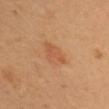Imaged during a routine full-body skin examination; the lesion was not biopsied and no histopathology is available. A female patient, aged 38–42. Captured under cross-polarized illumination. Cropped from a whole-body photographic skin survey; the tile spans about 15 mm. Longest diameter approximately 4 mm. Located on the head or neck.